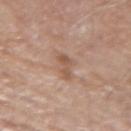The lesion was photographed on a routine skin check and not biopsied; there is no pathology result.
Located on the left forearm.
A 15 mm crop from a total-body photograph taken for skin-cancer surveillance.
The tile uses white-light illumination.
Longest diameter approximately 3 mm.
Automated tile analysis of the lesion measured a mean CIELAB color near L≈54 a*≈19 b*≈29 and a normalized lesion–skin contrast near 6.5. It also reported an automated nevus-likeness rating near 0 out of 100 and lesion-presence confidence of about 100/100.
A male patient aged around 70.